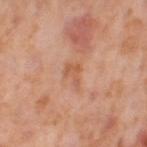The lesion was photographed on a routine skin check and not biopsied; there is no pathology result.
The lesion is on the right thigh.
This is a cross-polarized tile.
The lesion's longest dimension is about 3 mm.
A female subject about 55 years old.
This image is a 15 mm lesion crop taken from a total-body photograph.